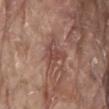No biopsy was performed on this lesion — it was imaged during a full skin examination and was not determined to be concerning.
On the lower back.
An algorithmic analysis of the crop reported a footprint of about 6.5 mm² and an eccentricity of roughly 0.65. The software also gave roughly 8 lightness units darker than nearby skin and a normalized lesion–skin contrast near 6. The software also gave a classifier nevus-likeness of about 0/100 and a lesion-detection confidence of about 90/100.
This image is a 15 mm lesion crop taken from a total-body photograph.
A male patient, about 80 years old.
Measured at roughly 3.5 mm in maximum diameter.
Imaged with white-light lighting.